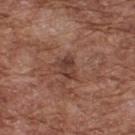workup = total-body-photography surveillance lesion; no biopsy | imaging modality = 15 mm crop, total-body photography | TBP lesion metrics = a mean CIELAB color near L≈39 a*≈21 b*≈25 and a lesion–skin lightness drop of about 9; a within-lesion color-variation index near 3.5/10 and peripheral color asymmetry of about 1.5 | body site = the upper back | lesion diameter = about 3 mm | tile lighting = white-light | patient = male, aged around 75.